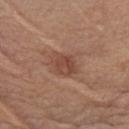Clinical impression:
Recorded during total-body skin imaging; not selected for excision or biopsy.
Image and clinical context:
The subject is a female in their mid- to late 60s. Measured at roughly 3.5 mm in maximum diameter. Cropped from a total-body skin-imaging series; the visible field is about 15 mm. The lesion is on the chest. Captured under white-light illumination. The total-body-photography lesion software estimated a lesion area of about 7.5 mm², an outline eccentricity of about 0.75 (0 = round, 1 = elongated), and a symmetry-axis asymmetry near 0.15.